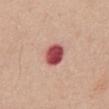follow-up: total-body-photography surveillance lesion; no biopsy | anatomic site: the abdomen | lesion diameter: ~3 mm (longest diameter) | imaging modality: 15 mm crop, total-body photography | subject: male, aged approximately 65.